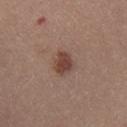An algorithmic analysis of the crop reported a mean CIELAB color near L≈43 a*≈20 b*≈24 and a lesion-to-skin contrast of about 8.5 (normalized; higher = more distinct).
Approximately 3 mm at its widest.
Located on the upper back.
This is a white-light tile.
Cropped from a whole-body photographic skin survey; the tile spans about 15 mm.
A female subject in their mid-20s.